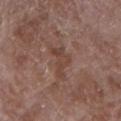Q: How large is the lesion?
A: ~4 mm (longest diameter)
Q: What did automated image analysis measure?
A: a lesion area of about 6.5 mm², a shape eccentricity near 0.8, and two-axis asymmetry of about 0.55; lesion-presence confidence of about 95/100
Q: Who is the patient?
A: female, aged around 70
Q: Lesion location?
A: the right upper arm
Q: How was this image acquired?
A: total-body-photography crop, ~15 mm field of view